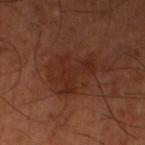{
  "biopsy_status": "not biopsied; imaged during a skin examination",
  "site": "left thigh",
  "patient": {
    "sex": "male",
    "age_approx": 65
  },
  "automated_metrics": {
    "nevus_likeness_0_100": 0,
    "lesion_detection_confidence_0_100": 100
  },
  "image": {
    "source": "total-body photography crop",
    "field_of_view_mm": 15
  },
  "lighting": "cross-polarized",
  "lesion_size": {
    "long_diameter_mm_approx": 5.5
  }
}